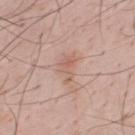The lesion was photographed on a routine skin check and not biopsied; there is no pathology result.
Imaged with white-light lighting.
The lesion's longest dimension is about 3.5 mm.
On the mid back.
A male patient aged around 35.
A region of skin cropped from a whole-body photographic capture, roughly 15 mm wide.